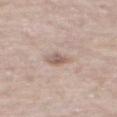Q: Was this lesion biopsied?
A: no biopsy performed (imaged during a skin exam)